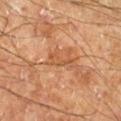Part of a total-body skin-imaging series; this lesion was reviewed on a skin check and was not flagged for biopsy. A roughly 15 mm field-of-view crop from a total-body skin photograph. The subject is a male about 60 years old. The lesion is located on the leg. The lesion's longest dimension is about 3.5 mm. Imaged with cross-polarized lighting.